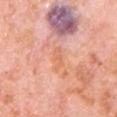Impression: No biopsy was performed on this lesion — it was imaged during a full skin examination and was not determined to be concerning. Acquisition and patient details: A close-up tile cropped from a whole-body skin photograph, about 15 mm across. Imaged with white-light lighting. The lesion's longest dimension is about 3 mm. A male subject aged 78–82. The lesion is on the chest.